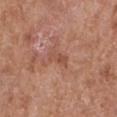{
  "biopsy_status": "not biopsied; imaged during a skin examination"
}